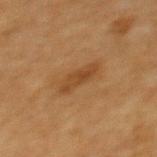No biopsy was performed on this lesion — it was imaged during a full skin examination and was not determined to be concerning. A close-up tile cropped from a whole-body skin photograph, about 15 mm across. From the upper back. The lesion's longest dimension is about 4 mm. The subject is a female in their mid-50s.